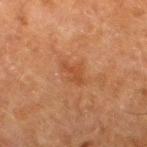The lesion was tiled from a total-body skin photograph and was not biopsied. On the right thigh. A male patient in their 80s. A close-up tile cropped from a whole-body skin photograph, about 15 mm across. Automated tile analysis of the lesion measured an average lesion color of about L≈38 a*≈21 b*≈31 (CIELAB), a lesion–skin lightness drop of about 6, and a normalized lesion–skin contrast near 5.5. The analysis additionally found a border-irregularity index near 3.5/10, a color-variation rating of about 1/10, and radial color variation of about 0. It also reported lesion-presence confidence of about 100/100. Approximately 3.5 mm at its widest.